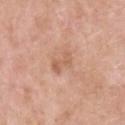Notes:
- biopsy status · imaged on a skin check; not biopsied
- diameter · about 3.5 mm
- automated lesion analysis · a lesion color around L≈60 a*≈22 b*≈32 in CIELAB, a lesion–skin lightness drop of about 8, and a normalized lesion–skin contrast near 5.5; a border-irregularity rating of about 4.5/10, internal color variation of about 2.5 on a 0–10 scale, and radial color variation of about 0.5; a lesion-detection confidence of about 100/100
- lighting · white-light
- acquisition · total-body-photography crop, ~15 mm field of view
- location · the upper back
- patient · male, aged 53 to 57